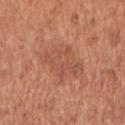Automated tile analysis of the lesion measured an eccentricity of roughly 0.8 and a symmetry-axis asymmetry near 0.3. It also reported a mean CIELAB color near L≈53 a*≈25 b*≈32. A female patient roughly 50 years of age. Captured under white-light illumination. Longest diameter approximately 6 mm. A 15 mm crop from a total-body photograph taken for skin-cancer surveillance. Located on the right upper arm.